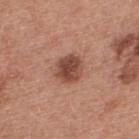* biopsy status · no biopsy performed (imaged during a skin exam)
* site · the upper back
* tile lighting · white-light
* patient · male, aged around 30
* image · 15 mm crop, total-body photography